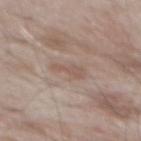Q: Was this lesion biopsied?
A: catalogued during a skin exam; not biopsied
Q: What kind of image is this?
A: ~15 mm crop, total-body skin-cancer survey
Q: What is the anatomic site?
A: the mid back
Q: What is the lesion's diameter?
A: about 3 mm
Q: Patient demographics?
A: male, approximately 55 years of age